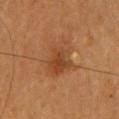follow-up = no biopsy performed (imaged during a skin exam)
acquisition = ~15 mm crop, total-body skin-cancer survey
diameter = ≈4 mm
subject = female, approximately 60 years of age
site = the upper back